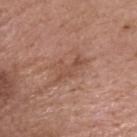About 4.5 mm across.
A male subject approximately 50 years of age.
A 15 mm close-up extracted from a 3D total-body photography capture.
The lesion is on the head or neck.
Imaged with white-light lighting.